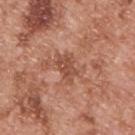{
  "biopsy_status": "not biopsied; imaged during a skin examination",
  "lighting": "white-light",
  "site": "upper back",
  "lesion_size": {
    "long_diameter_mm_approx": 4.0
  },
  "image": {
    "source": "total-body photography crop",
    "field_of_view_mm": 15
  },
  "patient": {
    "sex": "male",
    "age_approx": 55
  },
  "automated_metrics": {
    "nevus_likeness_0_100": 0,
    "lesion_detection_confidence_0_100": 100
  }
}